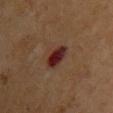<case>
  <biopsy_status>not biopsied; imaged during a skin examination</biopsy_status>
  <lighting>cross-polarized</lighting>
  <image>
    <source>total-body photography crop</source>
    <field_of_view_mm>15</field_of_view_mm>
  </image>
  <lesion_size>
    <long_diameter_mm_approx>3.5</long_diameter_mm_approx>
  </lesion_size>
  <automated_metrics>
    <vs_skin_darker_L>12.0</vs_skin_darker_L>
    <vs_skin_contrast_norm>13.5</vs_skin_contrast_norm>
    <border_irregularity_0_10>2.0</border_irregularity_0_10>
    <color_variation_0_10>5.5</color_variation_0_10>
    <peripheral_color_asymmetry>2.0</peripheral_color_asymmetry>
  </automated_metrics>
  <site>chest</site>
  <patient>
    <sex>female</sex>
    <age_approx>55</age_approx>
  </patient>
</case>